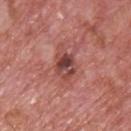<lesion>
  <biopsy_status>not biopsied; imaged during a skin examination</biopsy_status>
  <automated_metrics>
    <nevus_likeness_0_100>0</nevus_likeness_0_100>
    <lesion_detection_confidence_0_100>100</lesion_detection_confidence_0_100>
  </automated_metrics>
  <patient>
    <sex>male</sex>
    <age_approx>75</age_approx>
  </patient>
  <site>upper back</site>
  <image>
    <source>total-body photography crop</source>
    <field_of_view_mm>15</field_of_view_mm>
  </image>
  <lesion_size>
    <long_diameter_mm_approx>3.5</long_diameter_mm_approx>
  </lesion_size>
</lesion>